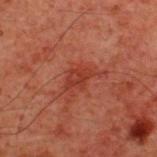Context:
The lesion's longest dimension is about 3 mm. The lesion is located on the upper back. A 15 mm close-up tile from a total-body photography series done for melanoma screening. Imaged with cross-polarized lighting. A male patient, in their 60s.